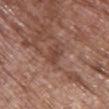Imaged during a routine full-body skin examination; the lesion was not biopsied and no histopathology is available. The tile uses white-light illumination. A female patient aged approximately 65. A 15 mm crop from a total-body photograph taken for skin-cancer surveillance. The lesion-visualizer software estimated a mean CIELAB color near L≈43 a*≈21 b*≈27, about 7 CIELAB-L* units darker than the surrounding skin, and a lesion-to-skin contrast of about 6 (normalized; higher = more distinct). Approximately 3 mm at its widest. From the front of the torso.